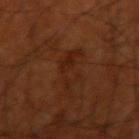Q: Is there a histopathology result?
A: no biopsy performed (imaged during a skin exam)
Q: What kind of image is this?
A: 15 mm crop, total-body photography
Q: Illumination type?
A: cross-polarized illumination
Q: How large is the lesion?
A: ≈5 mm
Q: What are the patient's age and sex?
A: male, about 70 years old
Q: Where on the body is the lesion?
A: the right upper arm